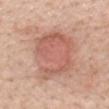The lesion was tiled from a total-body skin photograph and was not biopsied. Approximately 6.5 mm at its widest. The subject is a female aged 48–52. On the mid back. Cropped from a whole-body photographic skin survey; the tile spans about 15 mm. Imaged with white-light lighting.